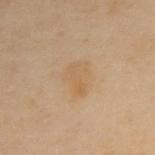workup: total-body-photography surveillance lesion; no biopsy
automated lesion analysis: a mean CIELAB color near L≈52 a*≈13 b*≈32, roughly 4 lightness units darker than nearby skin, and a normalized border contrast of about 5; a border-irregularity index near 2.5/10, a within-lesion color-variation index near 3/10, and radial color variation of about 1
lesion diameter: about 3.5 mm
image: 15 mm crop, total-body photography
subject: female, in their 60s
site: the upper back